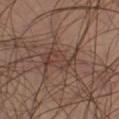No biopsy was performed on this lesion — it was imaged during a full skin examination and was not determined to be concerning.
The recorded lesion diameter is about 5 mm.
A male subject approximately 40 years of age.
The lesion is located on the right lower leg.
A close-up tile cropped from a whole-body skin photograph, about 15 mm across.